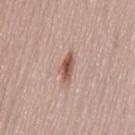Imaged during a routine full-body skin examination; the lesion was not biopsied and no histopathology is available. The lesion is on the lower back. A roughly 15 mm field-of-view crop from a total-body skin photograph. The subject is a female about 50 years old.